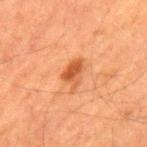Findings:
• notes · total-body-photography surveillance lesion; no biopsy
• site · the back
• lesion diameter · about 3.5 mm
• lighting · cross-polarized illumination
• image · ~15 mm crop, total-body skin-cancer survey
• image-analysis metrics · a classifier nevus-likeness of about 85/100 and a lesion-detection confidence of about 100/100
• subject · male, aged 58–62